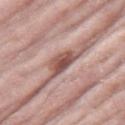The lesion was tiled from a total-body skin photograph and was not biopsied.
On the right thigh.
Captured under white-light illumination.
Automated image analysis of the tile measured a footprint of about 5 mm², a shape eccentricity near 0.8, and a shape-asymmetry score of about 0.3 (0 = symmetric). And it measured a nevus-likeness score of about 0/100.
A female subject about 70 years old.
About 3 mm across.
A 15 mm close-up extracted from a 3D total-body photography capture.